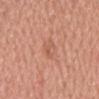Recorded during total-body skin imaging; not selected for excision or biopsy.
Longest diameter approximately 3 mm.
The lesion is on the head or neck.
This image is a 15 mm lesion crop taken from a total-body photograph.
The total-body-photography lesion software estimated a border-irregularity index near 3.5/10, a within-lesion color-variation index near 3/10, and radial color variation of about 1.
A male subject in their 50s.